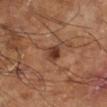  biopsy_status: not biopsied; imaged during a skin examination
  site: right lower leg
  patient:
    sex: male
    age_approx: 65
  automated_metrics:
    area_mm2_approx: 4.5
    eccentricity: 0.4
    shape_asymmetry: 0.25
    cielab_L: 39
    cielab_a: 21
    cielab_b: 29
    vs_skin_darker_L: 11.0
    nevus_likeness_0_100: 85
    lesion_detection_confidence_0_100: 100
  image:
    source: total-body photography crop
    field_of_view_mm: 15